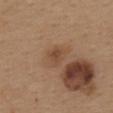<record>
  <biopsy_status>not biopsied; imaged during a skin examination</biopsy_status>
  <patient>
    <sex>female</sex>
    <age_approx>40</age_approx>
  </patient>
  <site>upper back</site>
  <automated_metrics>
    <area_mm2_approx>4.5</area_mm2_approx>
    <eccentricity>0.45</eccentricity>
    <shape_asymmetry>0.3</shape_asymmetry>
    <border_irregularity_0_10>3.0</border_irregularity_0_10>
    <color_variation_0_10>3.0</color_variation_0_10>
    <peripheral_color_asymmetry>1.0</peripheral_color_asymmetry>
  </automated_metrics>
  <lighting>white-light</lighting>
  <lesion_size>
    <long_diameter_mm_approx>2.5</long_diameter_mm_approx>
  </lesion_size>
  <image>
    <source>total-body photography crop</source>
    <field_of_view_mm>15</field_of_view_mm>
  </image>
</record>